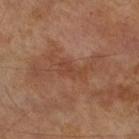Part of a total-body skin-imaging series; this lesion was reviewed on a skin check and was not flagged for biopsy.
Cropped from a whole-body photographic skin survey; the tile spans about 15 mm.
The lesion is on the leg.
A male patient aged approximately 70.